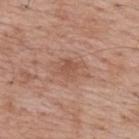Case summary:
• notes — no biopsy performed (imaged during a skin exam)
• lesion diameter — ~4 mm (longest diameter)
• lighting — white-light
• patient — male, aged 68–72
• image — total-body-photography crop, ~15 mm field of view
• TBP lesion metrics — a lesion color around L≈54 a*≈21 b*≈29 in CIELAB, about 8 CIELAB-L* units darker than the surrounding skin, and a normalized border contrast of about 5.5; a nevus-likeness score of about 15/100 and a lesion-detection confidence of about 100/100
• site — the upper back